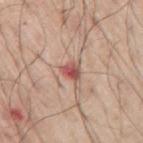Notes:
• workup — no biopsy performed (imaged during a skin exam)
• lighting — white-light illumination
• image — total-body-photography crop, ~15 mm field of view
• patient — male, aged 68–72
• automated lesion analysis — a footprint of about 4 mm² and two-axis asymmetry of about 0.35; a border-irregularity index near 3/10, a within-lesion color-variation index near 8.5/10, and peripheral color asymmetry of about 3.5; a classifier nevus-likeness of about 0/100
• site — the arm
• lesion size — ≈2.5 mm